A female subject aged 23 to 27. Longest diameter approximately 8.5 mm. The lesion is located on the abdomen. A close-up tile cropped from a whole-body skin photograph, about 15 mm across: 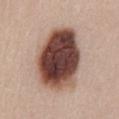Histopathological examination showed a dysplastic (Clark) nevus — a benign skin lesion.